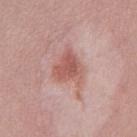Findings:
- notes · no biopsy performed (imaged during a skin exam)
- size · about 4 mm
- patient · female, about 35 years old
- illumination · white-light
- acquisition · 15 mm crop, total-body photography
- location · the chest